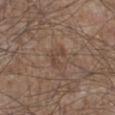notes: imaged on a skin check; not biopsied | image: ~15 mm crop, total-body skin-cancer survey | patient: male, approximately 65 years of age | site: the left lower leg | automated metrics: a lesion color around L≈44 a*≈16 b*≈25 in CIELAB, roughly 6 lightness units darker than nearby skin, and a normalized border contrast of about 5; a nevus-likeness score of about 0/100 and a lesion-detection confidence of about 100/100 | diameter: ≈2.5 mm.